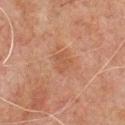Context:
Cropped from a total-body skin-imaging series; the visible field is about 15 mm. From the front of the torso. The tile uses cross-polarized illumination. A male patient, aged 58–62. Automated tile analysis of the lesion measured a mean CIELAB color near L≈40 a*≈18 b*≈27, about 5 CIELAB-L* units darker than the surrounding skin, and a normalized border contrast of about 4.5. It also reported a nevus-likeness score of about 0/100 and lesion-presence confidence of about 100/100. Measured at roughly 2.5 mm in maximum diameter.